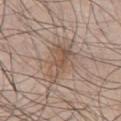Assessment: No biopsy was performed on this lesion — it was imaged during a full skin examination and was not determined to be concerning. Context: On the chest. The subject is a male in their mid-50s. Longest diameter approximately 5.5 mm. A 15 mm crop from a total-body photograph taken for skin-cancer surveillance. Imaged with white-light lighting.